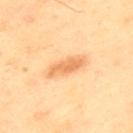workup — catalogued during a skin exam; not biopsied
tile lighting — cross-polarized illumination
lesion size — about 4.5 mm
subject — male, approximately 40 years of age
imaging modality — ~15 mm tile from a whole-body skin photo
site — the upper back